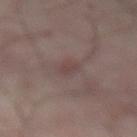{"biopsy_status": "not biopsied; imaged during a skin examination", "lighting": "cross-polarized", "patient": {"sex": "male", "age_approx": 50}, "automated_metrics": {"area_mm2_approx": 3.0, "eccentricity": 0.85, "shape_asymmetry": 0.35, "cielab_L": 41, "cielab_a": 16, "cielab_b": 17, "vs_skin_contrast_norm": 5.0}, "site": "abdomen", "image": {"source": "total-body photography crop", "field_of_view_mm": 15}}